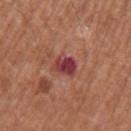Impression: Recorded during total-body skin imaging; not selected for excision or biopsy. Clinical summary: The recorded lesion diameter is about 3 mm. A male subject aged approximately 65. On the left upper arm. A lesion tile, about 15 mm wide, cut from a 3D total-body photograph.